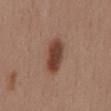The lesion was photographed on a routine skin check and not biopsied; there is no pathology result.
This is a white-light tile.
The lesion-visualizer software estimated a lesion color around L≈42 a*≈21 b*≈27 in CIELAB, about 13 CIELAB-L* units darker than the surrounding skin, and a lesion-to-skin contrast of about 10.5 (normalized; higher = more distinct). And it measured border irregularity of about 2 on a 0–10 scale and radial color variation of about 1.
A female patient roughly 55 years of age.
A lesion tile, about 15 mm wide, cut from a 3D total-body photograph.
On the mid back.